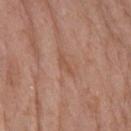  biopsy_status: not biopsied; imaged during a skin examination
  site: right forearm
  lighting: white-light
  lesion_size:
    long_diameter_mm_approx: 2.5
  patient:
    sex: female
    age_approx: 60
  image:
    source: total-body photography crop
    field_of_view_mm: 15